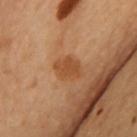Imaged during a routine full-body skin examination; the lesion was not biopsied and no histopathology is available. Automated tile analysis of the lesion measured a footprint of about 6.5 mm², a shape eccentricity near 0.55, and a shape-asymmetry score of about 0.15 (0 = symmetric). It also reported border irregularity of about 2 on a 0–10 scale, internal color variation of about 2 on a 0–10 scale, and radial color variation of about 0.5. A lesion tile, about 15 mm wide, cut from a 3D total-body photograph. The lesion is located on the chest. The tile uses cross-polarized illumination. Measured at roughly 3.5 mm in maximum diameter. The subject is a female about 60 years old.